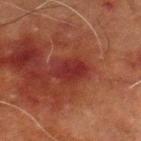workup: no biopsy performed (imaged during a skin exam) | size: ≈4 mm | TBP lesion metrics: a footprint of about 7 mm² and a shape-asymmetry score of about 0.2 (0 = symmetric); border irregularity of about 2 on a 0–10 scale, a within-lesion color-variation index near 2.5/10, and radial color variation of about 1; an automated nevus-likeness rating near 15 out of 100 and a lesion-detection confidence of about 100/100 | body site: the left lower leg | lighting: cross-polarized | image: ~15 mm crop, total-body skin-cancer survey | subject: male, aged 68–72.